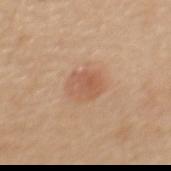The lesion was photographed on a routine skin check and not biopsied; there is no pathology result. The patient is a female approximately 55 years of age. The lesion is on the upper back. Measured at roughly 4 mm in maximum diameter. The tile uses white-light illumination. The total-body-photography lesion software estimated an outline eccentricity of about 0.6 (0 = round, 1 = elongated). And it measured border irregularity of about 1.5 on a 0–10 scale and a color-variation rating of about 3.5/10. And it measured a lesion-detection confidence of about 100/100. Cropped from a total-body skin-imaging series; the visible field is about 15 mm.